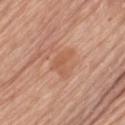Captured during whole-body skin photography for melanoma surveillance; the lesion was not biopsied. A female subject, aged around 75. Captured under white-light illumination. A 15 mm close-up extracted from a 3D total-body photography capture. The lesion is located on the left thigh.